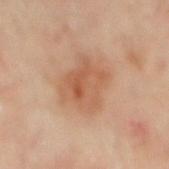workup: total-body-photography surveillance lesion; no biopsy
patient: male, in their mid-60s
body site: the mid back
diameter: about 5 mm
imaging modality: 15 mm crop, total-body photography
automated lesion analysis: a lesion area of about 17 mm², an outline eccentricity of about 0.55 (0 = round, 1 = elongated), and a shape-asymmetry score of about 0.25 (0 = symmetric); a mean CIELAB color near L≈56 a*≈21 b*≈33 and a normalized border contrast of about 6; an automated nevus-likeness rating near 65 out of 100